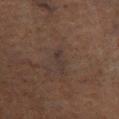Assessment:
The lesion was tiled from a total-body skin photograph and was not biopsied.
Acquisition and patient details:
Cropped from a whole-body photographic skin survey; the tile spans about 15 mm. Longest diameter approximately 3 mm. From the left lower leg. The tile uses cross-polarized illumination. A male patient, aged around 75.